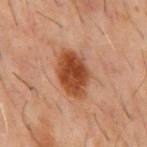* follow-up — no biopsy performed (imaged during a skin exam)
* patient — male, in their 60s
* illumination — cross-polarized illumination
* automated lesion analysis — a footprint of about 14 mm², a shape eccentricity near 0.8, and two-axis asymmetry of about 0.15; about 14 CIELAB-L* units darker than the surrounding skin
* body site — the mid back
* diameter — ≈5.5 mm
* image source — ~15 mm crop, total-body skin-cancer survey